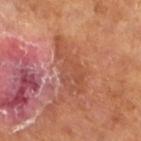Notes:
• biopsy status · imaged on a skin check; not biopsied
• lesion size · ~6.5 mm (longest diameter)
• image source · total-body-photography crop, ~15 mm field of view
• subject · male, roughly 65 years of age
• tile lighting · cross-polarized